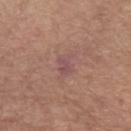No biopsy was performed on this lesion — it was imaged during a full skin examination and was not determined to be concerning.